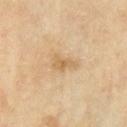Part of a total-body skin-imaging series; this lesion was reviewed on a skin check and was not flagged for biopsy.
A region of skin cropped from a whole-body photographic capture, roughly 15 mm wide.
A male patient in their mid-60s.
From the right upper arm.